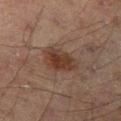TBP lesion metrics=border irregularity of about 2.5 on a 0–10 scale, internal color variation of about 3.5 on a 0–10 scale, and peripheral color asymmetry of about 1 | image source=total-body-photography crop, ~15 mm field of view | diameter=≈4.5 mm | site=the left thigh | lighting=cross-polarized | subject=male, aged around 70.